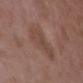| feature | finding |
|---|---|
| follow-up | total-body-photography surveillance lesion; no biopsy |
| image-analysis metrics | an area of roughly 15 mm² and a shape eccentricity near 0.8; a mean CIELAB color near L≈45 a*≈18 b*≈24 and about 6 CIELAB-L* units darker than the surrounding skin; a classifier nevus-likeness of about 0/100 and lesion-presence confidence of about 100/100 |
| location | the back |
| patient | female, aged around 35 |
| lesion size | ≈5.5 mm |
| image | 15 mm crop, total-body photography |
| lighting | white-light |